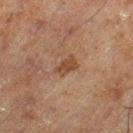  biopsy_status: not biopsied; imaged during a skin examination
  automated_metrics:
    shape_asymmetry: 0.3
    nevus_likeness_0_100: 45
    lesion_detection_confidence_0_100: 100
  lesion_size:
    long_diameter_mm_approx: 2.5
  site: left thigh
  image:
    source: total-body photography crop
    field_of_view_mm: 15
  patient:
    sex: male
    age_approx: 70